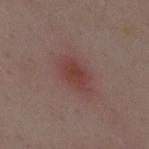Notes:
– biopsy status — no biopsy performed (imaged during a skin exam)
– image — ~15 mm tile from a whole-body skin photo
– subject — female, aged 33–37
– lesion size — about 4 mm
– TBP lesion metrics — a lesion area of about 7 mm² and a shape-asymmetry score of about 0.2 (0 = symmetric); a lesion–skin lightness drop of about 8 and a normalized border contrast of about 7; a nevus-likeness score of about 80/100 and lesion-presence confidence of about 100/100
– lighting — white-light
– anatomic site — the back